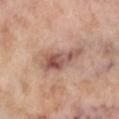workup: no biopsy performed (imaged during a skin exam)
anatomic site: the left lower leg
subject: female, in their mid-50s
image-analysis metrics: a lesion area of about 11 mm² and an outline eccentricity of about 0.95 (0 = round, 1 = elongated); a border-irregularity rating of about 5/10, internal color variation of about 8 on a 0–10 scale, and peripheral color asymmetry of about 2.5
image: 15 mm crop, total-body photography
lighting: cross-polarized
diameter: about 6.5 mm